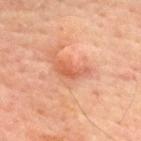notes: no biopsy performed (imaged during a skin exam)
diameter: ≈4 mm
TBP lesion metrics: a peripheral color-asymmetry measure near 0.5
image: ~15 mm crop, total-body skin-cancer survey
location: the chest
tile lighting: cross-polarized
subject: male, aged 68–72